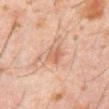<case>
<biopsy_status>not biopsied; imaged during a skin examination</biopsy_status>
<patient>
  <sex>male</sex>
  <age_approx>60</age_approx>
</patient>
<lighting>cross-polarized</lighting>
<site>abdomen</site>
<image>
  <source>total-body photography crop</source>
  <field_of_view_mm>15</field_of_view_mm>
</image>
<automated_metrics>
  <cielab_L>58</cielab_L>
  <cielab_a>22</cielab_a>
  <cielab_b>31</cielab_b>
  <border_irregularity_0_10>2.5</border_irregularity_0_10>
  <peripheral_color_asymmetry>1.0</peripheral_color_asymmetry>
</automated_metrics>
<lesion_size>
  <long_diameter_mm_approx>2.5</long_diameter_mm_approx>
</lesion_size>
</case>